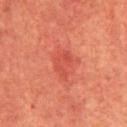Part of a total-body skin-imaging series; this lesion was reviewed on a skin check and was not flagged for biopsy.
The patient is a male in their mid-60s.
Cropped from a whole-body photographic skin survey; the tile spans about 15 mm.
The lesion's longest dimension is about 3.5 mm.
This is a cross-polarized tile.
From the chest.